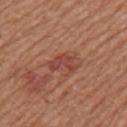biopsy_status: not biopsied; imaged during a skin examination
automated_metrics:
  border_irregularity_0_10: 4.0
  color_variation_0_10: 2.5
  peripheral_color_asymmetry: 1.0
  nevus_likeness_0_100: 35
  lesion_detection_confidence_0_100: 100
patient:
  sex: male
  age_approx: 55
site: left upper arm
image:
  source: total-body photography crop
  field_of_view_mm: 15
lesion_size:
  long_diameter_mm_approx: 4.0